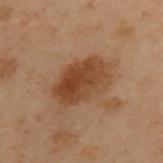Context: A male subject in their mid- to late 50s. The tile uses cross-polarized illumination. The lesion is located on the back. Automated image analysis of the tile measured a mean CIELAB color near L≈35 a*≈18 b*≈28, roughly 9 lightness units darker than nearby skin, and a normalized border contrast of about 8.5. The lesion's longest dimension is about 6 mm. A 15 mm close-up extracted from a 3D total-body photography capture.